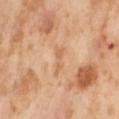Q: Was this lesion biopsied?
A: no biopsy performed (imaged during a skin exam)
Q: What kind of image is this?
A: ~15 mm crop, total-body skin-cancer survey
Q: What is the anatomic site?
A: the abdomen
Q: Illumination type?
A: cross-polarized illumination
Q: Who is the patient?
A: female, about 55 years old